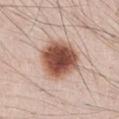<record>
  <biopsy_status>not biopsied; imaged during a skin examination</biopsy_status>
  <lesion_size>
    <long_diameter_mm_approx>5.0</long_diameter_mm_approx>
  </lesion_size>
  <patient>
    <sex>male</sex>
    <age_approx>35</age_approx>
  </patient>
  <image>
    <source>total-body photography crop</source>
    <field_of_view_mm>15</field_of_view_mm>
  </image>
  <site>abdomen</site>
  <automated_metrics>
    <cielab_L>52</cielab_L>
    <cielab_a>22</cielab_a>
    <cielab_b>28</cielab_b>
    <vs_skin_contrast_norm>13.0</vs_skin_contrast_norm>
    <border_irregularity_0_10>1.5</border_irregularity_0_10>
    <color_variation_0_10>6.5</color_variation_0_10>
    <peripheral_color_asymmetry>1.5</peripheral_color_asymmetry>
  </automated_metrics>
</record>